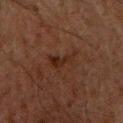follow-up: total-body-photography surveillance lesion; no biopsy
site: the chest
size: about 3.5 mm
patient: male, roughly 50 years of age
imaging modality: ~15 mm crop, total-body skin-cancer survey